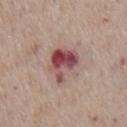The lesion was photographed on a routine skin check and not biopsied; there is no pathology result. This is a white-light tile. Longest diameter approximately 4 mm. A 15 mm crop from a total-body photograph taken for skin-cancer surveillance. The subject is a male aged approximately 75. Located on the front of the torso.